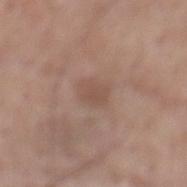Imaged during a routine full-body skin examination; the lesion was not biopsied and no histopathology is available.
A roughly 15 mm field-of-view crop from a total-body skin photograph.
The lesion's longest dimension is about 3 mm.
A male patient aged 58 to 62.
Automated image analysis of the tile measured a footprint of about 5 mm², an eccentricity of roughly 0.6, and two-axis asymmetry of about 0.2. The software also gave a classifier nevus-likeness of about 0/100 and a detector confidence of about 100 out of 100 that the crop contains a lesion.
Located on the mid back.
The tile uses white-light illumination.